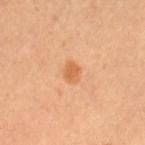Recorded during total-body skin imaging; not selected for excision or biopsy. A 15 mm close-up tile from a total-body photography series done for melanoma screening. The lesion is located on the leg. This is a cross-polarized tile. The patient is a female aged around 35. Longest diameter approximately 2 mm.